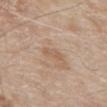notes: imaged on a skin check; not biopsied
anatomic site: the front of the torso
image source: ~15 mm tile from a whole-body skin photo
patient: male, aged 78–82